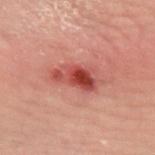Findings:
• biopsy status: catalogued during a skin exam; not biopsied
• site: the left forearm
• subject: male, roughly 40 years of age
• imaging modality: total-body-photography crop, ~15 mm field of view
• illumination: cross-polarized